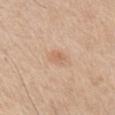– subject: male, in their mid- to late 60s
– image-analysis metrics: a mean CIELAB color near L≈64 a*≈20 b*≈32, roughly 7 lightness units darker than nearby skin, and a lesion-to-skin contrast of about 5 (normalized; higher = more distinct); a border-irregularity index near 1.5/10, internal color variation of about 1.5 on a 0–10 scale, and radial color variation of about 0.5
– tile lighting: white-light illumination
– site: the chest
– image source: 15 mm crop, total-body photography